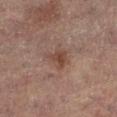Assessment: The lesion was photographed on a routine skin check and not biopsied; there is no pathology result. Background: Measured at roughly 3 mm in maximum diameter. Cropped from a total-body skin-imaging series; the visible field is about 15 mm. Captured under cross-polarized illumination. The total-body-photography lesion software estimated a border-irregularity index near 3.5/10, internal color variation of about 3 on a 0–10 scale, and radial color variation of about 1. The subject is a female in their 80s. The lesion is on the right leg.